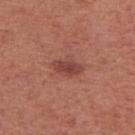This lesion was catalogued during total-body skin photography and was not selected for biopsy. The lesion is on the right upper arm. This image is a 15 mm lesion crop taken from a total-body photograph. A female patient, roughly 50 years of age.